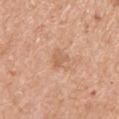Q: Was this lesion biopsied?
A: total-body-photography surveillance lesion; no biopsy
Q: Illumination type?
A: white-light
Q: Where on the body is the lesion?
A: the arm
Q: Patient demographics?
A: female, in their 70s
Q: What kind of image is this?
A: ~15 mm crop, total-body skin-cancer survey